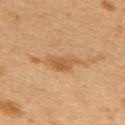Recorded during total-body skin imaging; not selected for excision or biopsy. The lesion-visualizer software estimated a lesion area of about 7 mm², an outline eccentricity of about 0.85 (0 = round, 1 = elongated), and a shape-asymmetry score of about 0.4 (0 = symmetric). Approximately 4.5 mm at its widest. A lesion tile, about 15 mm wide, cut from a 3D total-body photograph. The patient is a female in their 40s. From the upper back.